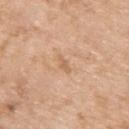notes: no biopsy performed (imaged during a skin exam); anatomic site: the right upper arm; lighting: white-light; image: ~15 mm tile from a whole-body skin photo; patient: female, aged 73 to 77.